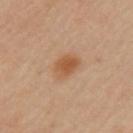Impression:
This lesion was catalogued during total-body skin photography and was not selected for biopsy.
Context:
This image is a 15 mm lesion crop taken from a total-body photograph. Imaged with cross-polarized lighting. On the left upper arm. Longest diameter approximately 3.5 mm. A female subject aged approximately 40.